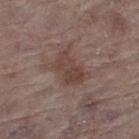Captured during whole-body skin photography for melanoma surveillance; the lesion was not biopsied. The subject is a female aged around 85 or older. The lesion is located on the leg. A close-up tile cropped from a whole-body skin photograph, about 15 mm across.